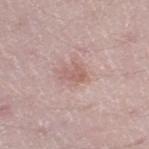Clinical impression:
Imaged during a routine full-body skin examination; the lesion was not biopsied and no histopathology is available.
Image and clinical context:
A male subject aged approximately 50. A roughly 15 mm field-of-view crop from a total-body skin photograph. The lesion is located on the left thigh. Captured under white-light illumination. Approximately 2.5 mm at its widest. The total-body-photography lesion software estimated border irregularity of about 4.5 on a 0–10 scale, internal color variation of about 1.5 on a 0–10 scale, and peripheral color asymmetry of about 0.5.